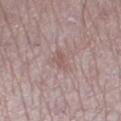The lesion was photographed on a routine skin check and not biopsied; there is no pathology result.
The patient is a female in their mid-60s.
A roughly 15 mm field-of-view crop from a total-body skin photograph.
The lesion is on the leg.